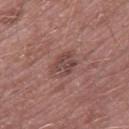{
  "biopsy_status": "not biopsied; imaged during a skin examination",
  "patient": {
    "sex": "male",
    "age_approx": 55
  },
  "site": "right thigh",
  "image": {
    "source": "total-body photography crop",
    "field_of_view_mm": 15
  }
}